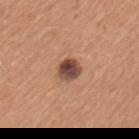Assessment: This lesion was catalogued during total-body skin photography and was not selected for biopsy. Image and clinical context: Located on the left upper arm. A female subject aged 28 to 32. A region of skin cropped from a whole-body photographic capture, roughly 15 mm wide. The lesion's longest dimension is about 2.5 mm.